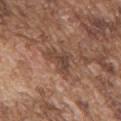This lesion was catalogued during total-body skin photography and was not selected for biopsy.
A male subject aged 73 to 77.
The tile uses white-light illumination.
Located on the upper back.
A roughly 15 mm field-of-view crop from a total-body skin photograph.